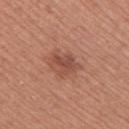biopsy status = catalogued during a skin exam; not biopsied
subject = female, aged 38 to 42
acquisition = ~15 mm tile from a whole-body skin photo
site = the right upper arm